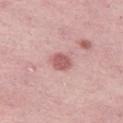<tbp_lesion>
<biopsy_status>not biopsied; imaged during a skin examination</biopsy_status>
<patient>
  <sex>female</sex>
  <age_approx>45</age_approx>
</patient>
<lighting>white-light</lighting>
<site>left thigh</site>
<image>
  <source>total-body photography crop</source>
  <field_of_view_mm>15</field_of_view_mm>
</image>
</tbp_lesion>